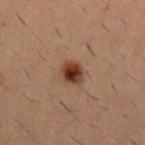{
  "biopsy_status": "not biopsied; imaged during a skin examination",
  "lesion_size": {
    "long_diameter_mm_approx": 2.5
  },
  "site": "right upper arm",
  "image": {
    "source": "total-body photography crop",
    "field_of_view_mm": 15
  },
  "patient": {
    "sex": "male",
    "age_approx": 30
  }
}